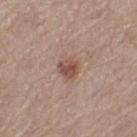follow-up: imaged on a skin check; not biopsied | subject: female, about 40 years old | tile lighting: white-light illumination | location: the left thigh | diameter: ~2.5 mm (longest diameter) | imaging modality: 15 mm crop, total-body photography | TBP lesion metrics: a shape eccentricity near 0.4; a lesion color around L≈50 a*≈19 b*≈26 in CIELAB and a lesion-to-skin contrast of about 8 (normalized; higher = more distinct); a color-variation rating of about 2.5/10 and radial color variation of about 1; a nevus-likeness score of about 75/100 and a lesion-detection confidence of about 100/100.